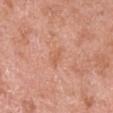No biopsy was performed on this lesion — it was imaged during a full skin examination and was not determined to be concerning.